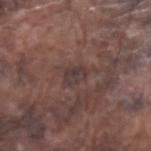Recorded during total-body skin imaging; not selected for excision or biopsy.
The lesion's longest dimension is about 2.5 mm.
This image is a 15 mm lesion crop taken from a total-body photograph.
Located on the left forearm.
Imaged with white-light lighting.
The subject is a male aged around 75.